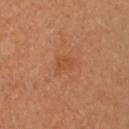Recorded during total-body skin imaging; not selected for excision or biopsy. A female subject. The lesion is located on the upper back. Automated tile analysis of the lesion measured an eccentricity of roughly 0.7 and two-axis asymmetry of about 0.3. And it measured a mean CIELAB color near L≈52 a*≈26 b*≈38, a lesion–skin lightness drop of about 5, and a lesion-to-skin contrast of about 4.5 (normalized; higher = more distinct). And it measured a nevus-likeness score of about 0/100 and a lesion-detection confidence of about 100/100. This is a cross-polarized tile. A region of skin cropped from a whole-body photographic capture, roughly 15 mm wide. The lesion's longest dimension is about 3 mm.